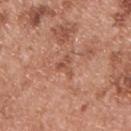Findings:
* follow-up: no biopsy performed (imaged during a skin exam)
* anatomic site: the upper back
* patient: male, about 55 years old
* acquisition: 15 mm crop, total-body photography